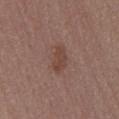Recorded during total-body skin imaging; not selected for excision or biopsy.